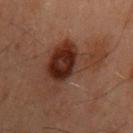  biopsy_status: not biopsied; imaged during a skin examination
  automated_metrics:
    area_mm2_approx: 25.0
    eccentricity: 0.75
    shape_asymmetry: 0.55
    cielab_L: 24
    cielab_a: 16
    cielab_b: 21
    vs_skin_darker_L: 8.0
    vs_skin_contrast_norm: 9.5
    lesion_detection_confidence_0_100: 100
  patient:
    sex: male
    age_approx: 60
  image:
    source: total-body photography crop
    field_of_view_mm: 15
  lesion_size:
    long_diameter_mm_approx: 8.0
  site: mid back